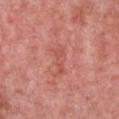* notes — imaged on a skin check; not biopsied
* patient — female, aged 38–42
* location — the chest
* image source — 15 mm crop, total-body photography